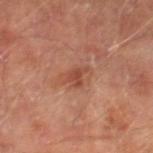| key | value |
|---|---|
| follow-up | no biopsy performed (imaged during a skin exam) |
| image-analysis metrics | an area of roughly 3.5 mm², an eccentricity of roughly 0.65, and a symmetry-axis asymmetry near 0.45; a nevus-likeness score of about 5/100 |
| illumination | cross-polarized |
| imaging modality | 15 mm crop, total-body photography |
| anatomic site | the leg |
| subject | male, in their mid-60s |